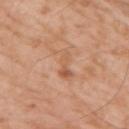Case summary:
• biopsy status: total-body-photography surveillance lesion; no biopsy
• image: ~15 mm tile from a whole-body skin photo
• body site: the right upper arm
• subject: male, in their 60s
• diameter: ≈4.5 mm